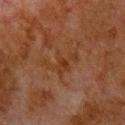Background:
The recorded lesion diameter is about 5 mm. Captured under cross-polarized illumination. A 15 mm crop from a total-body photograph taken for skin-cancer surveillance. The lesion is on the front of the torso. The patient is a male aged around 80.